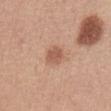Automated tile analysis of the lesion measured a shape eccentricity near 0.5.
The subject is a female in their mid-50s.
The lesion's longest dimension is about 2.5 mm.
On the abdomen.
A 15 mm close-up extracted from a 3D total-body photography capture.